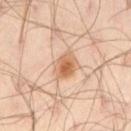* biopsy status — imaged on a skin check; not biopsied
* tile lighting — cross-polarized
* patient — male, aged approximately 45
* body site — the right thigh
* image source — 15 mm crop, total-body photography
* diameter — ~3 mm (longest diameter)